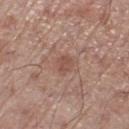<tbp_lesion>
<biopsy_status>not biopsied; imaged during a skin examination</biopsy_status>
<site>right lower leg</site>
<lesion_size>
  <long_diameter_mm_approx>2.5</long_diameter_mm_approx>
</lesion_size>
<image>
  <source>total-body photography crop</source>
  <field_of_view_mm>15</field_of_view_mm>
</image>
<patient>
  <sex>male</sex>
  <age_approx>70</age_approx>
</patient>
<lighting>white-light</lighting>
</tbp_lesion>